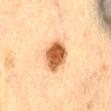Impression: The lesion was photographed on a routine skin check and not biopsied; there is no pathology result. Context: Imaged with cross-polarized lighting. On the lower back. The subject is a female aged 53–57. Measured at roughly 4.5 mm in maximum diameter. A 15 mm close-up tile from a total-body photography series done for melanoma screening.